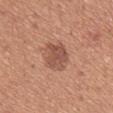Findings:
– notes — no biopsy performed (imaged during a skin exam)
– tile lighting — white-light
– lesion size — about 5 mm
– body site — the back
– image source — total-body-photography crop, ~15 mm field of view
– patient — female, about 50 years old